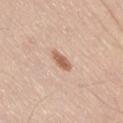<tbp_lesion>
<lesion_size>
  <long_diameter_mm_approx>3.0</long_diameter_mm_approx>
</lesion_size>
<patient>
  <sex>male</sex>
  <age_approx>40</age_approx>
</patient>
<site>leg</site>
<lighting>white-light</lighting>
<image>
  <source>total-body photography crop</source>
  <field_of_view_mm>15</field_of_view_mm>
</image>
</tbp_lesion>